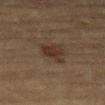biopsy status: catalogued during a skin exam; not biopsied
subject: male, about 85 years old or older
anatomic site: the leg
image: ~15 mm crop, total-body skin-cancer survey